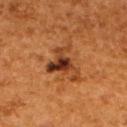Captured during whole-body skin photography for melanoma surveillance; the lesion was not biopsied. Captured under cross-polarized illumination. Cropped from a total-body skin-imaging series; the visible field is about 15 mm. A female subject aged around 50. The lesion is located on the back.